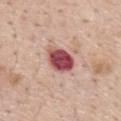Impression:
This lesion was catalogued during total-body skin photography and was not selected for biopsy.
Context:
The lesion-visualizer software estimated two-axis asymmetry of about 0.15. It also reported a border-irregularity rating of about 1.5/10, a color-variation rating of about 6/10, and a peripheral color-asymmetry measure near 2. A close-up tile cropped from a whole-body skin photograph, about 15 mm across. Located on the mid back. A male subject, in their mid- to late 50s. Longest diameter approximately 3.5 mm. The tile uses white-light illumination.